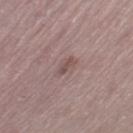Case summary:
– follow-up · imaged on a skin check; not biopsied
– automated metrics · an area of roughly 2 mm², an outline eccentricity of about 0.9 (0 = round, 1 = elongated), and a shape-asymmetry score of about 0.4 (0 = symmetric); a lesion color around L≈49 a*≈18 b*≈19 in CIELAB, about 8 CIELAB-L* units darker than the surrounding skin, and a lesion-to-skin contrast of about 6.5 (normalized; higher = more distinct); a nevus-likeness score of about 0/100 and a lesion-detection confidence of about 100/100
– acquisition · total-body-photography crop, ~15 mm field of view
– location · the left thigh
– subject · female, in their mid- to late 40s
– illumination · white-light illumination